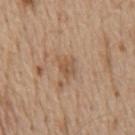Impression:
Imaged during a routine full-body skin examination; the lesion was not biopsied and no histopathology is available.
Context:
A roughly 15 mm field-of-view crop from a total-body skin photograph. The lesion's longest dimension is about 3 mm. A male subject approximately 70 years of age. This is a white-light tile. Located on the chest.